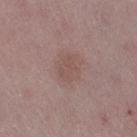Q: Is there a histopathology result?
A: imaged on a skin check; not biopsied
Q: How was the tile lit?
A: white-light illumination
Q: How large is the lesion?
A: ~3 mm (longest diameter)
Q: What is the imaging modality?
A: ~15 mm crop, total-body skin-cancer survey
Q: Who is the patient?
A: female, roughly 45 years of age
Q: Lesion location?
A: the right thigh